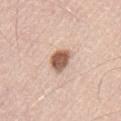| field | value |
|---|---|
| follow-up | no biopsy performed (imaged during a skin exam) |
| anatomic site | the left upper arm |
| acquisition | ~15 mm tile from a whole-body skin photo |
| patient | male, in their mid- to late 40s |